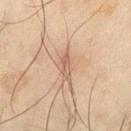Assessment:
This lesion was catalogued during total-body skin photography and was not selected for biopsy.
Acquisition and patient details:
A male patient aged around 45. A 15 mm crop from a total-body photograph taken for skin-cancer surveillance. The tile uses cross-polarized illumination. About 3.5 mm across. The lesion is on the left thigh.